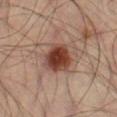This lesion was catalogued during total-body skin photography and was not selected for biopsy.
The lesion is on the right thigh.
A roughly 15 mm field-of-view crop from a total-body skin photograph.